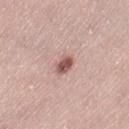follow-up — total-body-photography surveillance lesion; no biopsy | diameter — about 2.5 mm | TBP lesion metrics — a lesion area of about 3.5 mm², an eccentricity of roughly 0.75, and a symmetry-axis asymmetry near 0.2; border irregularity of about 2 on a 0–10 scale, a color-variation rating of about 2.5/10, and radial color variation of about 1; a classifier nevus-likeness of about 80/100 and a detector confidence of about 100 out of 100 that the crop contains a lesion | anatomic site — the left thigh | lighting — white-light illumination | image source — ~15 mm tile from a whole-body skin photo | subject — female, roughly 40 years of age.